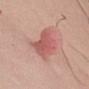| key | value |
|---|---|
| follow-up | no biopsy performed (imaged during a skin exam) |
| site | the right upper arm |
| image-analysis metrics | a lesion area of about 12 mm², an outline eccentricity of about 0.9 (0 = round, 1 = elongated), and two-axis asymmetry of about 0.35; roughly 11 lightness units darker than nearby skin; a color-variation rating of about 3/10 and a peripheral color-asymmetry measure near 1; an automated nevus-likeness rating near 10 out of 100 |
| subject | male, in their 50s |
| acquisition | ~15 mm crop, total-body skin-cancer survey |
| illumination | white-light |